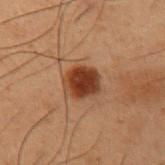Notes:
– workup — no biopsy performed (imaged during a skin exam)
– tile lighting — cross-polarized
– subject — male, about 55 years old
– lesion diameter — ~3.5 mm (longest diameter)
– location — the left upper arm
– acquisition — total-body-photography crop, ~15 mm field of view